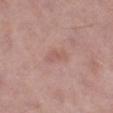Clinical impression:
Part of a total-body skin-imaging series; this lesion was reviewed on a skin check and was not flagged for biopsy.
Context:
Automated image analysis of the tile measured an area of roughly 3 mm², an outline eccentricity of about 0.85 (0 = round, 1 = elongated), and a shape-asymmetry score of about 0.35 (0 = symmetric). It also reported an average lesion color of about L≈57 a*≈22 b*≈25 (CIELAB), a lesion–skin lightness drop of about 6, and a lesion-to-skin contrast of about 4.5 (normalized; higher = more distinct). The software also gave a border-irregularity index near 3.5/10, a color-variation rating of about 0/10, and peripheral color asymmetry of about 0. The analysis additionally found an automated nevus-likeness rating near 0 out of 100. The lesion is on the left thigh. A region of skin cropped from a whole-body photographic capture, roughly 15 mm wide. This is a white-light tile. A male patient approximately 55 years of age. About 2.5 mm across.